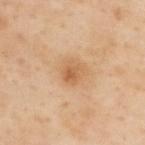Clinical impression: Imaged during a routine full-body skin examination; the lesion was not biopsied and no histopathology is available. Context: Imaged with cross-polarized lighting. A male subject, aged approximately 55. A 15 mm crop from a total-body photograph taken for skin-cancer surveillance. Automated image analysis of the tile measured a footprint of about 5 mm², an eccentricity of roughly 0.45, and a symmetry-axis asymmetry near 0.25. The lesion is located on the back. About 2.5 mm across.